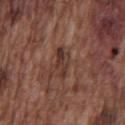No biopsy was performed on this lesion — it was imaged during a full skin examination and was not determined to be concerning.
The patient is a male about 75 years old.
The lesion-visualizer software estimated about 8 CIELAB-L* units darker than the surrounding skin. The software also gave border irregularity of about 3 on a 0–10 scale and internal color variation of about 5 on a 0–10 scale. It also reported a classifier nevus-likeness of about 0/100 and lesion-presence confidence of about 60/100.
Located on the front of the torso.
Measured at roughly 3.5 mm in maximum diameter.
This image is a 15 mm lesion crop taken from a total-body photograph.